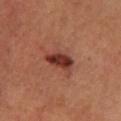{
  "biopsy_status": "not biopsied; imaged during a skin examination",
  "lighting": "cross-polarized",
  "patient": {
    "sex": "male",
    "age_approx": 55
  },
  "site": "leg",
  "lesion_size": {
    "long_diameter_mm_approx": 4.0
  },
  "image": {
    "source": "total-body photography crop",
    "field_of_view_mm": 15
  }
}